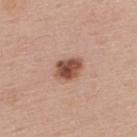Imaged during a routine full-body skin examination; the lesion was not biopsied and no histopathology is available.
The total-body-photography lesion software estimated an area of roughly 7 mm² and an outline eccentricity of about 0.7 (0 = round, 1 = elongated).
The lesion is on the upper back.
A male patient, aged 53 to 57.
A region of skin cropped from a whole-body photographic capture, roughly 15 mm wide.